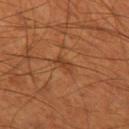The lesion was tiled from a total-body skin photograph and was not biopsied.
The patient is a male approximately 55 years of age.
The recorded lesion diameter is about 2.5 mm.
The tile uses cross-polarized illumination.
The lesion is located on the left thigh.
A close-up tile cropped from a whole-body skin photograph, about 15 mm across.